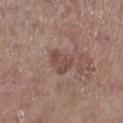From the left leg. A male patient roughly 80 years of age. The total-body-photography lesion software estimated a footprint of about 6 mm², an eccentricity of roughly 0.7, and a shape-asymmetry score of about 0.3 (0 = symmetric). Measured at roughly 3.5 mm in maximum diameter. A 15 mm crop from a total-body photograph taken for skin-cancer surveillance. Imaged with white-light lighting.